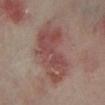Clinical impression:
Recorded during total-body skin imaging; not selected for excision or biopsy.
Context:
A region of skin cropped from a whole-body photographic capture, roughly 15 mm wide. The lesion is located on the left lower leg. The recorded lesion diameter is about 8.5 mm. The patient is a female aged around 35.